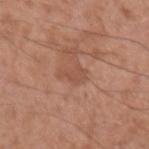Clinical impression:
Imaged during a routine full-body skin examination; the lesion was not biopsied and no histopathology is available.
Context:
Imaged with white-light lighting. A male patient, aged 48 to 52. A 15 mm close-up tile from a total-body photography series done for melanoma screening. The lesion is located on the left upper arm. The recorded lesion diameter is about 3 mm.